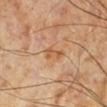Assessment: The lesion was tiled from a total-body skin photograph and was not biopsied. Clinical summary: A male patient, aged around 70. Cropped from a total-body skin-imaging series; the visible field is about 15 mm. The lesion is located on the leg. Imaged with cross-polarized lighting. Approximately 3 mm at its widest.